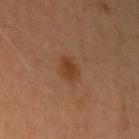Background:
The total-body-photography lesion software estimated a mean CIELAB color near L≈40 a*≈21 b*≈33 and a normalized lesion–skin contrast near 7. It also reported lesion-presence confidence of about 100/100. Cropped from a total-body skin-imaging series; the visible field is about 15 mm. The lesion is on the left upper arm. The tile uses cross-polarized illumination. The lesion's longest dimension is about 2.5 mm. A male patient, aged 38 to 42.